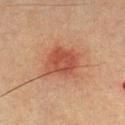* biopsy status: no biopsy performed (imaged during a skin exam)
* subject: male, roughly 40 years of age
* image: total-body-photography crop, ~15 mm field of view
* body site: the chest
* tile lighting: cross-polarized
* TBP lesion metrics: an automated nevus-likeness rating near 85 out of 100